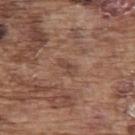notes: no biopsy performed (imaged during a skin exam)
lesion size: ≈2.5 mm
subject: male, in their mid-70s
body site: the upper back
image: ~15 mm tile from a whole-body skin photo
image-analysis metrics: a footprint of about 3 mm², an outline eccentricity of about 0.85 (0 = round, 1 = elongated), and a symmetry-axis asymmetry near 0.3; an average lesion color of about L≈45 a*≈19 b*≈26 (CIELAB) and a lesion-to-skin contrast of about 5.5 (normalized; higher = more distinct); internal color variation of about 1.5 on a 0–10 scale and radial color variation of about 0.5
tile lighting: white-light illumination